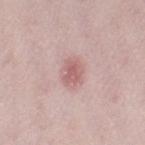workup: catalogued during a skin exam; not biopsied | size: about 3 mm | patient: female, aged around 30 | acquisition: 15 mm crop, total-body photography | automated lesion analysis: an average lesion color of about L≈60 a*≈23 b*≈21 (CIELAB) and a lesion–skin lightness drop of about 10; a border-irregularity index near 2.5/10, a color-variation rating of about 2/10, and peripheral color asymmetry of about 0.5; an automated nevus-likeness rating near 45 out of 100 and a lesion-detection confidence of about 100/100 | location: the left thigh.